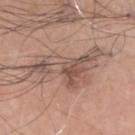body site = the head or neck
tile lighting = white-light illumination
image = 15 mm crop, total-body photography
lesion diameter = ≈9.5 mm
subject = male, about 65 years old